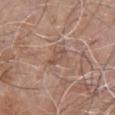The lesion was tiled from a total-body skin photograph and was not biopsied.
On the chest.
A male patient aged 78–82.
A region of skin cropped from a whole-body photographic capture, roughly 15 mm wide.